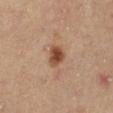Q: Was a biopsy performed?
A: no biopsy performed (imaged during a skin exam)
Q: What kind of image is this?
A: total-body-photography crop, ~15 mm field of view
Q: What did automated image analysis measure?
A: a footprint of about 4.5 mm², a shape eccentricity near 0.5, and a shape-asymmetry score of about 0.25 (0 = symmetric); an average lesion color of about L≈45 a*≈20 b*≈31 (CIELAB), a lesion–skin lightness drop of about 13, and a normalized border contrast of about 10; a border-irregularity index near 2/10 and a color-variation rating of about 3/10; a classifier nevus-likeness of about 95/100 and lesion-presence confidence of about 100/100
Q: What are the patient's age and sex?
A: female, aged approximately 45
Q: Lesion size?
A: ≈2.5 mm
Q: Where on the body is the lesion?
A: the left lower leg
Q: How was the tile lit?
A: cross-polarized